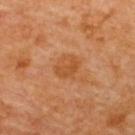Assessment:
Recorded during total-body skin imaging; not selected for excision or biopsy.
Acquisition and patient details:
A female subject, aged approximately 65. The tile uses cross-polarized illumination. Automated image analysis of the tile measured a symmetry-axis asymmetry near 0.15. The software also gave a border-irregularity index near 1.5/10, a within-lesion color-variation index near 2.5/10, and radial color variation of about 1. The software also gave a classifier nevus-likeness of about 10/100 and lesion-presence confidence of about 100/100. On the upper back. A lesion tile, about 15 mm wide, cut from a 3D total-body photograph.